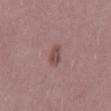• follow-up: total-body-photography surveillance lesion; no biopsy
• imaging modality: total-body-photography crop, ~15 mm field of view
• subject: male, aged approximately 50
• TBP lesion metrics: an area of roughly 3.5 mm², an eccentricity of roughly 0.85, and two-axis asymmetry of about 0.3; roughly 9 lightness units darker than nearby skin and a lesion-to-skin contrast of about 7.5 (normalized; higher = more distinct); border irregularity of about 3 on a 0–10 scale, a within-lesion color-variation index near 2.5/10, and peripheral color asymmetry of about 1
• location: the mid back
• lesion size: about 3 mm
• illumination: white-light